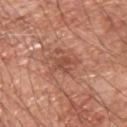No biopsy was performed on this lesion — it was imaged during a full skin examination and was not determined to be concerning. On the upper back. A male subject, aged 63–67. Captured under white-light illumination. The lesion's longest dimension is about 2.5 mm. Cropped from a total-body skin-imaging series; the visible field is about 15 mm.